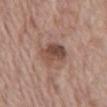From the front of the torso. A male patient aged 68–72. Captured under white-light illumination. A 15 mm close-up extracted from a 3D total-body photography capture. About 4 mm across.